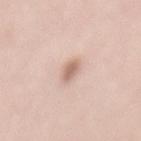Clinical impression:
Captured during whole-body skin photography for melanoma surveillance; the lesion was not biopsied.
Image and clinical context:
This is a white-light tile. The total-body-photography lesion software estimated a footprint of about 4 mm², an eccentricity of roughly 0.8, and two-axis asymmetry of about 0.3. It also reported a lesion color around L≈66 a*≈18 b*≈26 in CIELAB and a normalized border contrast of about 7. And it measured a border-irregularity rating of about 2.5/10 and a color-variation rating of about 2/10. The software also gave an automated nevus-likeness rating near 95 out of 100 and lesion-presence confidence of about 100/100. The recorded lesion diameter is about 3 mm. The subject is a female roughly 40 years of age. A close-up tile cropped from a whole-body skin photograph, about 15 mm across. The lesion is on the mid back.